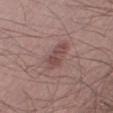The lesion was photographed on a routine skin check and not biopsied; there is no pathology result. A 15 mm crop from a total-body photograph taken for skin-cancer surveillance. Automated image analysis of the tile measured a mean CIELAB color near L≈47 a*≈21 b*≈20, a lesion–skin lightness drop of about 9, and a normalized border contrast of about 6.5. The analysis additionally found border irregularity of about 3.5 on a 0–10 scale and peripheral color asymmetry of about 0.5. The analysis additionally found a classifier nevus-likeness of about 10/100 and a detector confidence of about 100 out of 100 that the crop contains a lesion. The subject is a male in their mid-20s. The lesion is located on the right thigh. This is a white-light tile. The lesion's longest dimension is about 4 mm.